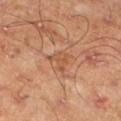The lesion was photographed on a routine skin check and not biopsied; there is no pathology result.
The lesion is located on the leg.
A male patient about 45 years old.
Measured at roughly 2.5 mm in maximum diameter.
Automated tile analysis of the lesion measured a lesion area of about 4 mm². The software also gave border irregularity of about 5.5 on a 0–10 scale, a color-variation rating of about 2/10, and a peripheral color-asymmetry measure near 0.5.
A 15 mm close-up tile from a total-body photography series done for melanoma screening.